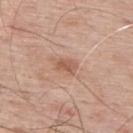{
  "biopsy_status": "not biopsied; imaged during a skin examination",
  "patient": {
    "sex": "male",
    "age_approx": 65
  },
  "lighting": "white-light",
  "automated_metrics": {
    "area_mm2_approx": 4.0,
    "eccentricity": 0.8,
    "shape_asymmetry": 0.25,
    "cielab_L": 57,
    "cielab_a": 22,
    "cielab_b": 30,
    "vs_skin_darker_L": 9.0,
    "vs_skin_contrast_norm": 6.5,
    "border_irregularity_0_10": 2.5,
    "color_variation_0_10": 3.5,
    "peripheral_color_asymmetry": 1.5,
    "nevus_likeness_0_100": 15,
    "lesion_detection_confidence_0_100": 100
  },
  "lesion_size": {
    "long_diameter_mm_approx": 2.5
  },
  "image": {
    "source": "total-body photography crop",
    "field_of_view_mm": 15
  },
  "site": "upper back"
}